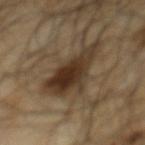follow-up=catalogued during a skin exam; not biopsied | size=≈6.5 mm | body site=the back | lighting=cross-polarized | subject=male, roughly 65 years of age | imaging modality=~15 mm tile from a whole-body skin photo.